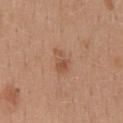The lesion was tiled from a total-body skin photograph and was not biopsied.